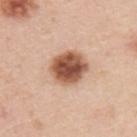Captured during whole-body skin photography for melanoma surveillance; the lesion was not biopsied. Cropped from a whole-body photographic skin survey; the tile spans about 15 mm. The tile uses white-light illumination. From the upper back. A male patient aged around 45.